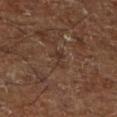Case summary:
• follow-up · imaged on a skin check; not biopsied
• image source · total-body-photography crop, ~15 mm field of view
• subject · aged approximately 65
• site · the right lower leg
• illumination · cross-polarized illumination
• lesion diameter · ~3 mm (longest diameter)